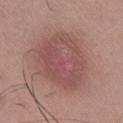{
  "biopsy_status": "not biopsied; imaged during a skin examination",
  "automated_metrics": {
    "area_mm2_approx": 33.0,
    "shape_asymmetry": 0.15,
    "cielab_L": 50,
    "cielab_a": 23,
    "cielab_b": 21,
    "vs_skin_contrast_norm": 6.0,
    "border_irregularity_0_10": 2.0,
    "color_variation_0_10": 4.0,
    "peripheral_color_asymmetry": 1.0,
    "lesion_detection_confidence_0_100": 100
  },
  "site": "right lower leg",
  "image": {
    "source": "total-body photography crop",
    "field_of_view_mm": 15
  },
  "lesion_size": {
    "long_diameter_mm_approx": 7.0
  },
  "lighting": "white-light",
  "patient": {
    "sex": "male",
    "age_approx": 35
  }
}